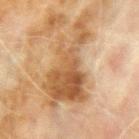<lesion>
  <biopsy_status>not biopsied; imaged during a skin examination</biopsy_status>
  <image>
    <source>total-body photography crop</source>
    <field_of_view_mm>15</field_of_view_mm>
  </image>
  <patient>
    <sex>male</sex>
    <age_approx>70</age_approx>
  </patient>
  <lighting>cross-polarized</lighting>
  <site>arm</site>
</lesion>